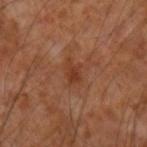| key | value |
|---|---|
| notes | no biopsy performed (imaged during a skin exam) |
| image | 15 mm crop, total-body photography |
| lesion diameter | about 3 mm |
| site | the left forearm |
| patient | male, in their mid-50s |
| lighting | cross-polarized illumination |